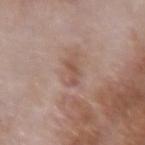  biopsy_status: not biopsied; imaged during a skin examination
  site: right forearm
  lighting: white-light
  patient:
    sex: male
    age_approx: 65
  image:
    source: total-body photography crop
    field_of_view_mm: 15
  lesion_size:
    long_diameter_mm_approx: 2.5